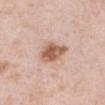Recorded during total-body skin imaging; not selected for excision or biopsy. On the right upper arm. Automated tile analysis of the lesion measured an area of roughly 8.5 mm², an eccentricity of roughly 0.65, and a symmetry-axis asymmetry near 0.25. It also reported a border-irregularity rating of about 2.5/10 and internal color variation of about 4.5 on a 0–10 scale. The subject is a male roughly 40 years of age. Cropped from a total-body skin-imaging series; the visible field is about 15 mm.